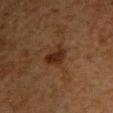workup: no biopsy performed (imaged during a skin exam)
subject: female, in their 60s
acquisition: ~15 mm crop, total-body skin-cancer survey
lighting: cross-polarized
body site: the upper back
TBP lesion metrics: an area of roughly 6 mm², an eccentricity of roughly 0.45, and a shape-asymmetry score of about 0.3 (0 = symmetric); a mean CIELAB color near L≈22 a*≈17 b*≈24, a lesion–skin lightness drop of about 7, and a normalized border contrast of about 8.5
diameter: ≈2.5 mm